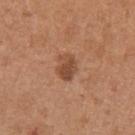Notes:
• biopsy status · catalogued during a skin exam; not biopsied
• patient · female, roughly 30 years of age
• lesion diameter · ≈3 mm
• image · ~15 mm tile from a whole-body skin photo
• site · the right upper arm
• lighting · white-light illumination